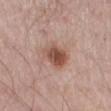Q: Is there a histopathology result?
A: imaged on a skin check; not biopsied